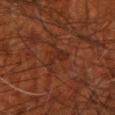Case summary:
- biopsy status — catalogued during a skin exam; not biopsied
- imaging modality — ~15 mm crop, total-body skin-cancer survey
- body site — the arm
- subject — male, aged around 70